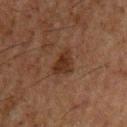  biopsy_status: not biopsied; imaged during a skin examination
  lesion_size:
    long_diameter_mm_approx: 3.5
  site: front of the torso
  patient:
    sex: male
    age_approx: 50
  image:
    source: total-body photography crop
    field_of_view_mm: 15